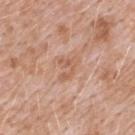follow-up: catalogued during a skin exam; not biopsied | image: 15 mm crop, total-body photography | patient: male, aged 48 to 52 | anatomic site: the upper back.